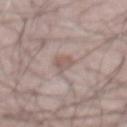Clinical impression: The lesion was tiled from a total-body skin photograph and was not biopsied. Clinical summary: About 2.5 mm across. A male patient, aged 63–67. A roughly 15 mm field-of-view crop from a total-body skin photograph. The lesion is located on the abdomen.